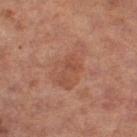{"biopsy_status": "not biopsied; imaged during a skin examination", "site": "right thigh", "automated_metrics": {"area_mm2_approx": 13.0, "eccentricity": 0.85, "shape_asymmetry": 0.4, "vs_skin_darker_L": 5.0, "vs_skin_contrast_norm": 4.5}, "lighting": "cross-polarized", "patient": {"sex": "female", "age_approx": 60}, "image": {"source": "total-body photography crop", "field_of_view_mm": 15}}